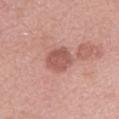Captured during whole-body skin photography for melanoma surveillance; the lesion was not biopsied.
This is a white-light tile.
A close-up tile cropped from a whole-body skin photograph, about 15 mm across.
About 3.5 mm across.
A female subject, in their 50s.
On the arm.
An algorithmic analysis of the crop reported an eccentricity of roughly 0.55 and two-axis asymmetry of about 0.2. The software also gave peripheral color asymmetry of about 1.